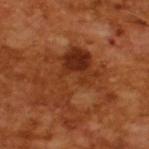Impression:
Recorded during total-body skin imaging; not selected for excision or biopsy.
Background:
The lesion-visualizer software estimated roughly 9 lightness units darker than nearby skin and a lesion-to-skin contrast of about 8 (normalized; higher = more distinct). It also reported a within-lesion color-variation index near 6.5/10 and radial color variation of about 2.5. It also reported a classifier nevus-likeness of about 0/100 and a detector confidence of about 100 out of 100 that the crop contains a lesion. Longest diameter approximately 7 mm. The subject is a male about 65 years old. This is a cross-polarized tile. Cropped from a whole-body photographic skin survey; the tile spans about 15 mm.